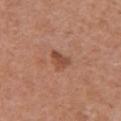- biopsy status · catalogued during a skin exam; not biopsied
- lighting · white-light
- patient · female, aged around 50
- body site · the chest
- image source · ~15 mm tile from a whole-body skin photo
- TBP lesion metrics · an area of roughly 4.5 mm², an eccentricity of roughly 0.7, and a symmetry-axis asymmetry near 0.45
- lesion diameter · about 3 mm